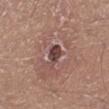Impression: This lesion was catalogued during total-body skin photography and was not selected for biopsy. Background: The recorded lesion diameter is about 3 mm. A male subject in their 30s. A lesion tile, about 15 mm wide, cut from a 3D total-body photograph. Captured under white-light illumination. The lesion is on the left lower leg.